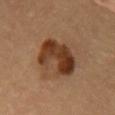Recorded during total-body skin imaging; not selected for excision or biopsy. The lesion is on the back. A lesion tile, about 15 mm wide, cut from a 3D total-body photograph. A male subject, aged around 60.